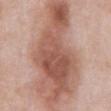Impression: No biopsy was performed on this lesion — it was imaged during a full skin examination and was not determined to be concerning. Acquisition and patient details: The patient is a male approximately 55 years of age. A close-up tile cropped from a whole-body skin photograph, about 15 mm across. The lesion is located on the front of the torso. Approximately 15 mm at its widest. The lesion-visualizer software estimated an area of roughly 65 mm², an outline eccentricity of about 0.9 (0 = round, 1 = elongated), and two-axis asymmetry of about 0.35. The analysis additionally found an automated nevus-likeness rating near 50 out of 100 and a lesion-detection confidence of about 100/100. Captured under white-light illumination.